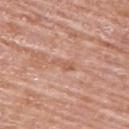Clinical impression: This lesion was catalogued during total-body skin photography and was not selected for biopsy. Background: This image is a 15 mm lesion crop taken from a total-body photograph. The patient is a female roughly 60 years of age. Located on the back. Imaged with white-light lighting. About 3 mm across.